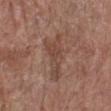Clinical impression:
Recorded during total-body skin imaging; not selected for excision or biopsy.
Image and clinical context:
Imaged with white-light lighting. An algorithmic analysis of the crop reported a mean CIELAB color near L≈44 a*≈20 b*≈26, about 7 CIELAB-L* units darker than the surrounding skin, and a normalized border contrast of about 6. It also reported a border-irregularity rating of about 8.5/10 and a color-variation rating of about 1.5/10. And it measured a nevus-likeness score of about 0/100 and a lesion-detection confidence of about 95/100. A roughly 15 mm field-of-view crop from a total-body skin photograph. The subject is a female in their 80s. The lesion is on the left lower leg. Measured at roughly 6 mm in maximum diameter.